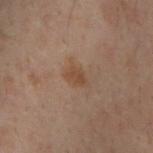Clinical impression:
No biopsy was performed on this lesion — it was imaged during a full skin examination and was not determined to be concerning.
Clinical summary:
About 3 mm across. This is a cross-polarized tile. From the upper back. A lesion tile, about 15 mm wide, cut from a 3D total-body photograph. The subject is a male aged 28 to 32.